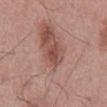Recorded during total-body skin imaging; not selected for excision or biopsy. On the mid back. About 3 mm across. The tile uses white-light illumination. A male subject aged 53 to 57. Cropped from a whole-body photographic skin survey; the tile spans about 15 mm.